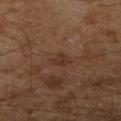  biopsy_status: not biopsied; imaged during a skin examination
  lesion_size:
    long_diameter_mm_approx: 2.5
  automated_metrics:
    area_mm2_approx: 3.5
    eccentricity: 0.7
    shape_asymmetry: 0.3
    vs_skin_darker_L: 6.0
    vs_skin_contrast_norm: 6.0
    color_variation_0_10: 2.0
    peripheral_color_asymmetry: 0.5
  image:
    source: total-body photography crop
    field_of_view_mm: 15
  patient:
    sex: male
    age_approx: 65